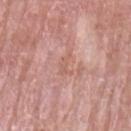workup = no biopsy performed (imaged during a skin exam) | image = ~15 mm crop, total-body skin-cancer survey | illumination = white-light | subject = female, aged approximately 60 | site = the left upper arm | TBP lesion metrics = a lesion area of about 3 mm², a shape eccentricity near 0.9, and two-axis asymmetry of about 0.65; a mean CIELAB color near L≈59 a*≈23 b*≈27, roughly 6 lightness units darker than nearby skin, and a normalized border contrast of about 5; a border-irregularity rating of about 8/10 and a color-variation rating of about 0/10; a lesion-detection confidence of about 60/100.